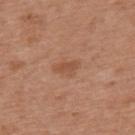Findings:
- notes: catalogued during a skin exam; not biopsied
- image-analysis metrics: two-axis asymmetry of about 0.3; an average lesion color of about L≈51 a*≈22 b*≈32 (CIELAB) and a normalized border contrast of about 5.5; a color-variation rating of about 1/10 and peripheral color asymmetry of about 0
- tile lighting: white-light
- acquisition: ~15 mm tile from a whole-body skin photo
- size: ~3 mm (longest diameter)
- site: the back
- subject: female, aged around 40